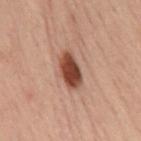<record>
  <biopsy_status>not biopsied; imaged during a skin examination</biopsy_status>
  <patient>
    <sex>female</sex>
    <age_approx>55</age_approx>
  </patient>
  <automated_metrics>
    <area_mm2_approx>9.0</area_mm2_approx>
    <eccentricity>0.85</eccentricity>
    <shape_asymmetry>0.2</shape_asymmetry>
    <cielab_L>38</cielab_L>
    <cielab_a>20</cielab_a>
    <cielab_b>25</cielab_b>
    <vs_skin_contrast_norm>12.0</vs_skin_contrast_norm>
    <lesion_detection_confidence_0_100>100</lesion_detection_confidence_0_100>
  </automated_metrics>
  <site>mid back</site>
  <image>
    <source>total-body photography crop</source>
    <field_of_view_mm>15</field_of_view_mm>
  </image>
  <lighting>cross-polarized</lighting>
</record>